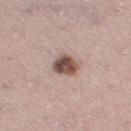image-analysis metrics = an average lesion color of about L≈50 a*≈19 b*≈22 (CIELAB) and a normalized border contrast of about 11.5; a border-irregularity index near 2/10 and peripheral color asymmetry of about 2 | patient = female, approximately 40 years of age | lesion diameter = ~3 mm (longest diameter) | anatomic site = the right thigh | image source = 15 mm crop, total-body photography | lighting = white-light.